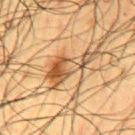follow-up — catalogued during a skin exam; not biopsied | lesion size — about 7 mm | anatomic site — the mid back | patient — male, aged 58–62 | imaging modality — 15 mm crop, total-body photography | automated lesion analysis — an outline eccentricity of about 0.95 (0 = round, 1 = elongated) and a symmetry-axis asymmetry near 0.55; a mean CIELAB color near L≈43 a*≈17 b*≈32, a lesion–skin lightness drop of about 11, and a lesion-to-skin contrast of about 9 (normalized; higher = more distinct); a border-irregularity index near 8/10, internal color variation of about 6.5 on a 0–10 scale, and peripheral color asymmetry of about 1.5.